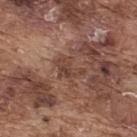Part of a total-body skin-imaging series; this lesion was reviewed on a skin check and was not flagged for biopsy. A male subject about 75 years old. A 15 mm close-up tile from a total-body photography series done for melanoma screening. The tile uses white-light illumination. Located on the back.